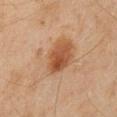Clinical impression: Part of a total-body skin-imaging series; this lesion was reviewed on a skin check and was not flagged for biopsy. Context: A 15 mm crop from a total-body photograph taken for skin-cancer surveillance. The tile uses cross-polarized illumination. An algorithmic analysis of the crop reported two-axis asymmetry of about 0.3. And it measured an average lesion color of about L≈45 a*≈17 b*≈29 (CIELAB), a lesion–skin lightness drop of about 7, and a lesion-to-skin contrast of about 6 (normalized; higher = more distinct). The analysis additionally found a border-irregularity index near 4.5/10, a within-lesion color-variation index near 6.5/10, and a peripheral color-asymmetry measure near 1.5. The analysis additionally found an automated nevus-likeness rating near 90 out of 100. A male patient aged around 45. From the mid back. The lesion's longest dimension is about 6 mm.